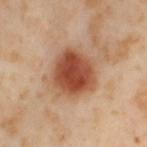Findings:
- notes — imaged on a skin check; not biopsied
- site — the left thigh
- illumination — cross-polarized
- image — total-body-photography crop, ~15 mm field of view
- subject — female, aged approximately 55